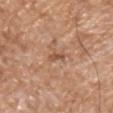Assessment:
Captured during whole-body skin photography for melanoma surveillance; the lesion was not biopsied.
Image and clinical context:
A 15 mm crop from a total-body photograph taken for skin-cancer surveillance. The lesion is located on the left upper arm. A male subject aged 63–67. Longest diameter approximately 2.5 mm.